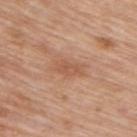The lesion was photographed on a routine skin check and not biopsied; there is no pathology result. This is a white-light tile. On the upper back. A male subject aged 53–57. The lesion-visualizer software estimated an area of roughly 5.5 mm², an outline eccentricity of about 0.9 (0 = round, 1 = elongated), and two-axis asymmetry of about 0.25. The analysis additionally found a lesion color around L≈56 a*≈22 b*≈33 in CIELAB and about 8 CIELAB-L* units darker than the surrounding skin. Cropped from a whole-body photographic skin survey; the tile spans about 15 mm.